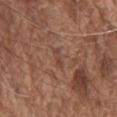Assessment:
The lesion was tiled from a total-body skin photograph and was not biopsied.
Acquisition and patient details:
The patient is a male in their mid- to late 70s. The recorded lesion diameter is about 2.5 mm. The lesion is located on the chest. A lesion tile, about 15 mm wide, cut from a 3D total-body photograph.